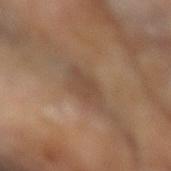{
  "biopsy_status": "not biopsied; imaged during a skin examination"
}